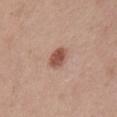workup: imaged on a skin check; not biopsied
subject: male, aged 23–27
anatomic site: the chest
acquisition: total-body-photography crop, ~15 mm field of view